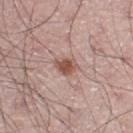{"biopsy_status": "not biopsied; imaged during a skin examination", "lighting": "white-light", "site": "right leg", "image": {"source": "total-body photography crop", "field_of_view_mm": 15}, "lesion_size": {"long_diameter_mm_approx": 3.0}, "patient": {"sex": "male", "age_approx": 70}}